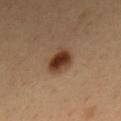Clinical impression: Imaged during a routine full-body skin examination; the lesion was not biopsied and no histopathology is available. Acquisition and patient details: The tile uses cross-polarized illumination. A close-up tile cropped from a whole-body skin photograph, about 15 mm across. The recorded lesion diameter is about 4 mm. A male subject, aged around 50. Located on the mid back. Automated tile analysis of the lesion measured a mean CIELAB color near L≈38 a*≈19 b*≈30 and about 14 CIELAB-L* units darker than the surrounding skin. It also reported an automated nevus-likeness rating near 100 out of 100 and lesion-presence confidence of about 100/100.